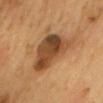Q: Is there a histopathology result?
A: catalogued during a skin exam; not biopsied
Q: Lesion location?
A: the chest
Q: What are the patient's age and sex?
A: male, in their mid-60s
Q: What is the lesion's diameter?
A: ≈8 mm
Q: What is the imaging modality?
A: ~15 mm crop, total-body skin-cancer survey
Q: What did automated image analysis measure?
A: a lesion area of about 21 mm² and a symmetry-axis asymmetry near 0.4; a border-irregularity index near 4.5/10 and a within-lesion color-variation index near 8/10; a nevus-likeness score of about 45/100
Q: What lighting was used for the tile?
A: cross-polarized illumination